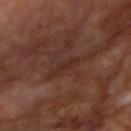Findings:
– notes · catalogued during a skin exam; not biopsied
– image · total-body-photography crop, ~15 mm field of view
– size · about 3 mm
– subject · male, aged 63–67
– image-analysis metrics · a lesion area of about 3 mm², an eccentricity of roughly 0.9, and a symmetry-axis asymmetry near 0.3; a border-irregularity rating of about 3.5/10, internal color variation of about 0.5 on a 0–10 scale, and radial color variation of about 0
– anatomic site · the arm
– tile lighting · cross-polarized